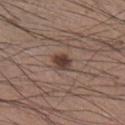Assessment: This lesion was catalogued during total-body skin photography and was not selected for biopsy. Background: A roughly 15 mm field-of-view crop from a total-body skin photograph. The recorded lesion diameter is about 2.5 mm. On the leg. Imaged with white-light lighting. A male patient in their mid-30s.